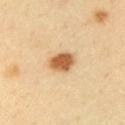No biopsy was performed on this lesion — it was imaged during a full skin examination and was not determined to be concerning. On the arm. A male subject, aged around 40. A lesion tile, about 15 mm wide, cut from a 3D total-body photograph.